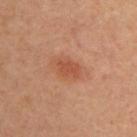Findings:
* workup · total-body-photography surveillance lesion; no biopsy
* image-analysis metrics · a lesion area of about 5.5 mm² and two-axis asymmetry of about 0.25; about 8 CIELAB-L* units darker than the surrounding skin and a normalized lesion–skin contrast near 6; border irregularity of about 2 on a 0–10 scale and internal color variation of about 1.5 on a 0–10 scale; a nevus-likeness score of about 80/100 and lesion-presence confidence of about 100/100
* location · the back
* acquisition · 15 mm crop, total-body photography
* patient · female, in their mid- to late 40s
* lesion diameter · about 3 mm